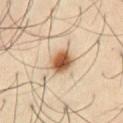<tbp_lesion>
  <biopsy_status>not biopsied; imaged during a skin examination</biopsy_status>
  <image>
    <source>total-body photography crop</source>
    <field_of_view_mm>15</field_of_view_mm>
  </image>
  <lighting>cross-polarized</lighting>
  <patient>
    <sex>male</sex>
    <age_approx>50</age_approx>
  </patient>
  <automated_metrics>
    <area_mm2_approx>7.5</area_mm2_approx>
    <eccentricity>0.6</eccentricity>
    <shape_asymmetry>0.15</shape_asymmetry>
    <border_irregularity_0_10>1.5</border_irregularity_0_10>
    <color_variation_0_10>7.0</color_variation_0_10>
    <peripheral_color_asymmetry>2.0</peripheral_color_asymmetry>
    <nevus_likeness_0_100>100</nevus_likeness_0_100>
    <lesion_detection_confidence_0_100>100</lesion_detection_confidence_0_100>
  </automated_metrics>
  <site>abdomen</site>
</tbp_lesion>